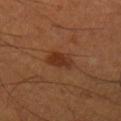* notes: catalogued during a skin exam; not biopsied